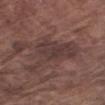Case summary:
– workup: total-body-photography surveillance lesion; no biopsy
– subject: male, aged 73 to 77
– location: the left upper arm
– imaging modality: 15 mm crop, total-body photography